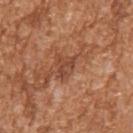– biopsy status · imaged on a skin check; not biopsied
– body site · the right upper arm
– TBP lesion metrics · a lesion area of about 2.5 mm² and an outline eccentricity of about 0.9 (0 = round, 1 = elongated); a lesion color around L≈44 a*≈24 b*≈32 in CIELAB and about 8 CIELAB-L* units darker than the surrounding skin; a border-irregularity index near 8/10, a color-variation rating of about 0/10, and peripheral color asymmetry of about 0
– size · ≈3 mm
– patient · male, approximately 45 years of age
– acquisition · ~15 mm crop, total-body skin-cancer survey
– tile lighting · white-light illumination